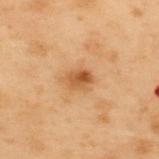<case>
<biopsy_status>not biopsied; imaged during a skin examination</biopsy_status>
<image>
  <source>total-body photography crop</source>
  <field_of_view_mm>15</field_of_view_mm>
</image>
<lesion_size>
  <long_diameter_mm_approx>2.5</long_diameter_mm_approx>
</lesion_size>
<site>upper back</site>
<lighting>cross-polarized</lighting>
<patient>
  <sex>male</sex>
  <age_approx>55</age_approx>
</patient>
<automated_metrics>
  <cielab_L>45</cielab_L>
  <cielab_a>20</cielab_a>
  <cielab_b>35</cielab_b>
  <vs_skin_darker_L>10.0</vs_skin_darker_L>
  <vs_skin_contrast_norm>8.0</vs_skin_contrast_norm>
</automated_metrics>
</case>